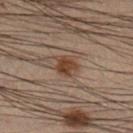Imaged during a routine full-body skin examination; the lesion was not biopsied and no histopathology is available.
A 15 mm crop from a total-body photograph taken for skin-cancer surveillance.
The recorded lesion diameter is about 3 mm.
The lesion is on the right lower leg.
A male patient aged 53–57.
The tile uses cross-polarized illumination.